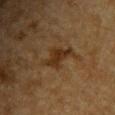Q: Was a biopsy performed?
A: no biopsy performed (imaged during a skin exam)
Q: How large is the lesion?
A: about 4 mm
Q: How was the tile lit?
A: cross-polarized
Q: What is the imaging modality?
A: total-body-photography crop, ~15 mm field of view
Q: Lesion location?
A: the upper back
Q: Patient demographics?
A: male, about 85 years old or older